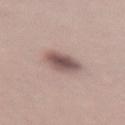Notes:
- notes — catalogued during a skin exam; not biopsied
- image — ~15 mm crop, total-body skin-cancer survey
- patient — male, aged approximately 45
- TBP lesion metrics — a normalized border contrast of about 9.5; a classifier nevus-likeness of about 60/100 and a lesion-detection confidence of about 100/100
- anatomic site — the right thigh
- tile lighting — white-light illumination